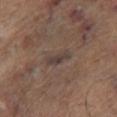This lesion was catalogued during total-body skin photography and was not selected for biopsy.